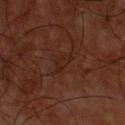Impression: The lesion was tiled from a total-body skin photograph and was not biopsied. Clinical summary: From the chest. The tile uses cross-polarized illumination. A male subject, aged around 60. A lesion tile, about 15 mm wide, cut from a 3D total-body photograph. Measured at roughly 3 mm in maximum diameter. An algorithmic analysis of the crop reported a border-irregularity rating of about 4/10, a within-lesion color-variation index near 1.5/10, and peripheral color asymmetry of about 0.5.